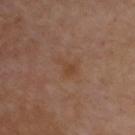follow-up: total-body-photography surveillance lesion; no biopsy
lighting: cross-polarized illumination
body site: the upper back
image: 15 mm crop, total-body photography
patient: male, aged 53–57
size: about 3 mm